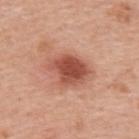Clinical impression:
Captured during whole-body skin photography for melanoma surveillance; the lesion was not biopsied.
Background:
A close-up tile cropped from a whole-body skin photograph, about 15 mm across. The lesion is on the upper back. The lesion-visualizer software estimated an outline eccentricity of about 0.7 (0 = round, 1 = elongated) and a shape-asymmetry score of about 0.2 (0 = symmetric). The software also gave a border-irregularity rating of about 2/10, a color-variation rating of about 3.5/10, and radial color variation of about 1. It also reported a classifier nevus-likeness of about 95/100 and lesion-presence confidence of about 100/100. Longest diameter approximately 5 mm. The patient is a male aged around 60. Captured under white-light illumination.